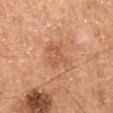No biopsy was performed on this lesion — it was imaged during a full skin examination and was not determined to be concerning.
An algorithmic analysis of the crop reported an area of roughly 4.5 mm² and an eccentricity of roughly 0.2. And it measured a mean CIELAB color near L≈54 a*≈24 b*≈34, about 7 CIELAB-L* units darker than the surrounding skin, and a normalized lesion–skin contrast near 5. And it measured border irregularity of about 9 on a 0–10 scale, internal color variation of about 0.5 on a 0–10 scale, and peripheral color asymmetry of about 0. The software also gave lesion-presence confidence of about 100/100.
A 15 mm crop from a total-body photograph taken for skin-cancer surveillance.
The tile uses cross-polarized illumination.
The recorded lesion diameter is about 3 mm.
Located on the leg.
The patient is a male roughly 60 years of age.